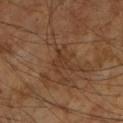follow-up: total-body-photography surveillance lesion; no biopsy.